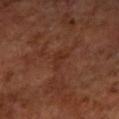location=the left forearm; lesion diameter=about 2.5 mm; acquisition=~15 mm tile from a whole-body skin photo; illumination=cross-polarized illumination; patient=female, approximately 65 years of age.